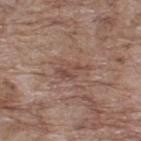notes — catalogued during a skin exam; not biopsied
acquisition — ~15 mm tile from a whole-body skin photo
lesion size — about 4 mm
location — the upper back
patient — male, aged 68 to 72